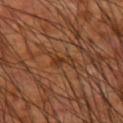follow-up: catalogued during a skin exam; not biopsied